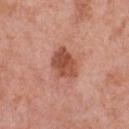Captured during whole-body skin photography for melanoma surveillance; the lesion was not biopsied.
A male subject, in their 70s.
On the chest.
A 15 mm close-up extracted from a 3D total-body photography capture.